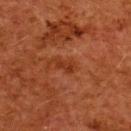{
  "biopsy_status": "not biopsied; imaged during a skin examination",
  "image": {
    "source": "total-body photography crop",
    "field_of_view_mm": 15
  },
  "site": "upper back",
  "patient": {
    "sex": "female",
    "age_approx": 50
  }
}